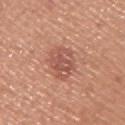{
  "biopsy_status": "not biopsied; imaged during a skin examination",
  "automated_metrics": {
    "border_irregularity_0_10": 2.0,
    "color_variation_0_10": 4.0,
    "nevus_likeness_0_100": 25
  },
  "patient": {
    "sex": "male",
    "age_approx": 45
  },
  "lesion_size": {
    "long_diameter_mm_approx": 4.0
  },
  "image": {
    "source": "total-body photography crop",
    "field_of_view_mm": 15
  },
  "lighting": "white-light",
  "site": "upper back"
}